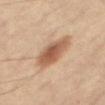Q: Who is the patient?
A: male, about 60 years old
Q: What is the lesion's diameter?
A: ≈6 mm
Q: What is the imaging modality?
A: ~15 mm tile from a whole-body skin photo
Q: How was the tile lit?
A: cross-polarized illumination
Q: Where on the body is the lesion?
A: the right thigh